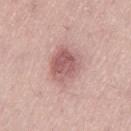Impression: The lesion was tiled from a total-body skin photograph and was not biopsied. Clinical summary: Imaged with white-light lighting. The lesion is located on the left thigh. A 15 mm close-up extracted from a 3D total-body photography capture. A female patient, in their 40s.